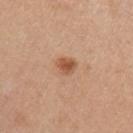biopsy status: imaged on a skin check; not biopsied
automated lesion analysis: an outline eccentricity of about 0.65 (0 = round, 1 = elongated)
site: the left upper arm
imaging modality: ~15 mm crop, total-body skin-cancer survey
lesion diameter: ≈2.5 mm
tile lighting: white-light
patient: female, about 65 years old